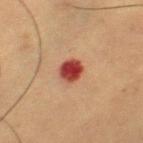| key | value |
|---|---|
| workup | catalogued during a skin exam; not biopsied |
| patient | male, approximately 55 years of age |
| body site | the leg |
| illumination | cross-polarized |
| image source | ~15 mm crop, total-body skin-cancer survey |